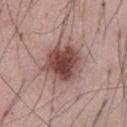{
  "site": "abdomen",
  "lesion_size": {
    "long_diameter_mm_approx": 5.0
  },
  "automated_metrics": {
    "area_mm2_approx": 18.0,
    "eccentricity": 0.35,
    "shape_asymmetry": 0.15,
    "cielab_L": 47,
    "cielab_a": 21,
    "cielab_b": 23,
    "vs_skin_darker_L": 15.0
  },
  "image": {
    "source": "total-body photography crop",
    "field_of_view_mm": 15
  },
  "patient": {
    "sex": "male",
    "age_approx": 55
  }
}